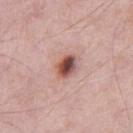Notes:
• notes — imaged on a skin check; not biopsied
• subject — male, aged 53–57
• tile lighting — white-light
• automated lesion analysis — an area of roughly 5 mm² and an outline eccentricity of about 0.65 (0 = round, 1 = elongated); a lesion color around L≈50 a*≈23 b*≈24 in CIELAB and roughly 18 lightness units darker than nearby skin; a border-irregularity index near 1.5/10, a within-lesion color-variation index near 8.5/10, and radial color variation of about 2.5
• location — the left thigh
• acquisition — total-body-photography crop, ~15 mm field of view
• lesion size — ~2.5 mm (longest diameter)